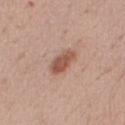Captured during whole-body skin photography for melanoma surveillance; the lesion was not biopsied. An algorithmic analysis of the crop reported a lesion–skin lightness drop of about 12 and a lesion-to-skin contrast of about 8.5 (normalized; higher = more distinct). The software also gave a border-irregularity rating of about 2/10, a within-lesion color-variation index near 3/10, and a peripheral color-asymmetry measure near 1. A 15 mm close-up tile from a total-body photography series done for melanoma screening. Longest diameter approximately 4 mm. The patient is a male aged 38–42. Captured under white-light illumination. From the right upper arm.